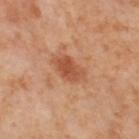  biopsy_status: not biopsied; imaged during a skin examination
  lesion_size:
    long_diameter_mm_approx: 3.5
  image:
    source: total-body photography crop
    field_of_view_mm: 15
  site: leg
  lighting: cross-polarized
  patient:
    sex: female
    age_approx: 55